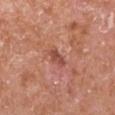notes = imaged on a skin check; not biopsied
anatomic site = the arm
patient = male, roughly 65 years of age
illumination = white-light
automated metrics = an area of roughly 3 mm² and two-axis asymmetry of about 0.3; border irregularity of about 3 on a 0–10 scale, a color-variation rating of about 2/10, and a peripheral color-asymmetry measure near 1; an automated nevus-likeness rating near 0 out of 100
size = ~2.5 mm (longest diameter)
imaging modality = ~15 mm crop, total-body skin-cancer survey